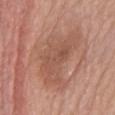Assessment:
Part of a total-body skin-imaging series; this lesion was reviewed on a skin check and was not flagged for biopsy.
Clinical summary:
A 15 mm crop from a total-body photograph taken for skin-cancer surveillance. A female subject about 75 years old. The lesion is located on the mid back. Automated image analysis of the tile measured a nevus-likeness score of about 0/100 and a lesion-detection confidence of about 100/100. This is a white-light tile. Approximately 8 mm at its widest.